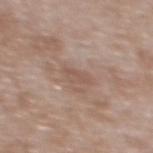Q: Was a biopsy performed?
A: no biopsy performed (imaged during a skin exam)
Q: What is the imaging modality?
A: total-body-photography crop, ~15 mm field of view
Q: How was the tile lit?
A: white-light
Q: Automated lesion metrics?
A: a lesion area of about 3.5 mm² and an eccentricity of roughly 0.85; an average lesion color of about L≈53 a*≈17 b*≈25 (CIELAB) and a lesion-to-skin contrast of about 4.5 (normalized; higher = more distinct); border irregularity of about 3 on a 0–10 scale, a within-lesion color-variation index near 1/10, and peripheral color asymmetry of about 0.5
Q: Lesion size?
A: ~3 mm (longest diameter)
Q: What are the patient's age and sex?
A: male, aged 48 to 52
Q: What is the anatomic site?
A: the mid back